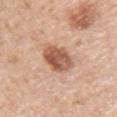| feature | finding |
|---|---|
| notes | catalogued during a skin exam; not biopsied |
| lighting | white-light illumination |
| subject | male, aged 38–42 |
| location | the arm |
| TBP lesion metrics | a border-irregularity rating of about 2/10, a color-variation rating of about 6/10, and peripheral color asymmetry of about 2 |
| image source | ~15 mm crop, total-body skin-cancer survey |